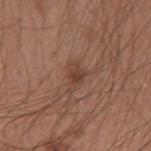<tbp_lesion>
  <lesion_size>
    <long_diameter_mm_approx>3.0</long_diameter_mm_approx>
  </lesion_size>
  <lighting>white-light</lighting>
  <patient>
    <sex>male</sex>
    <age_approx>30</age_approx>
  </patient>
  <site>left forearm</site>
  <automated_metrics>
    <eccentricity>0.7</eccentricity>
    <shape_asymmetry>0.45</shape_asymmetry>
    <vs_skin_darker_L>8.0</vs_skin_darker_L>
    <nevus_likeness_0_100>70</nevus_likeness_0_100>
  </automated_metrics>
  <image>
    <source>total-body photography crop</source>
    <field_of_view_mm>15</field_of_view_mm>
  </image>
</tbp_lesion>